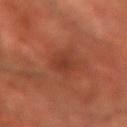Q: Was a biopsy performed?
A: imaged on a skin check; not biopsied
Q: Where on the body is the lesion?
A: the arm
Q: What is the imaging modality?
A: 15 mm crop, total-body photography
Q: Who is the patient?
A: male, aged 68–72